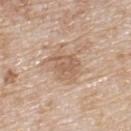follow-up = imaged on a skin check; not biopsied | acquisition = 15 mm crop, total-body photography | lesion size = ~3.5 mm (longest diameter) | body site = the upper back | patient = male, aged 78 to 82 | illumination = white-light illumination.